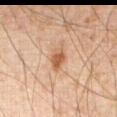workup=total-body-photography surveillance lesion; no biopsy | diameter=≈3 mm | site=the abdomen | acquisition=~15 mm crop, total-body skin-cancer survey | patient=male, roughly 65 years of age.